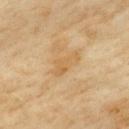{"biopsy_status": "not biopsied; imaged during a skin examination", "patient": {"sex": "female", "age_approx": 60}, "lighting": "cross-polarized", "site": "upper back", "lesion_size": {"long_diameter_mm_approx": 3.5}, "image": {"source": "total-body photography crop", "field_of_view_mm": 15}}